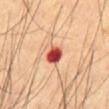Impression:
Part of a total-body skin-imaging series; this lesion was reviewed on a skin check and was not flagged for biopsy.
Image and clinical context:
From the front of the torso. A lesion tile, about 15 mm wide, cut from a 3D total-body photograph. Captured under cross-polarized illumination. The subject is a male aged 68–72. The recorded lesion diameter is about 2.5 mm.